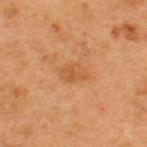No biopsy was performed on this lesion — it was imaged during a full skin examination and was not determined to be concerning.
From the upper back.
Longest diameter approximately 3 mm.
A female patient about 40 years old.
Captured under cross-polarized illumination.
A 15 mm crop from a total-body photograph taken for skin-cancer surveillance.